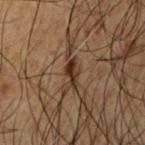Captured during whole-body skin photography for melanoma surveillance; the lesion was not biopsied. A 15 mm crop from a total-body photograph taken for skin-cancer surveillance. From the left upper arm. The subject is a male aged 48–52.